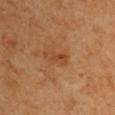Clinical impression:
This lesion was catalogued during total-body skin photography and was not selected for biopsy.